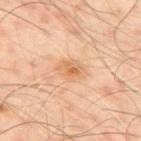follow-up = imaged on a skin check; not biopsied | image = 15 mm crop, total-body photography | lesion size = about 3 mm | subject = male, in their 50s | anatomic site = the upper back.